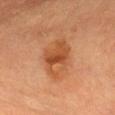Assessment: Recorded during total-body skin imaging; not selected for excision or biopsy.